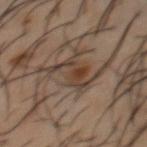workup: catalogued during a skin exam; not biopsied | patient: male, about 55 years old | size: ~5 mm (longest diameter) | image-analysis metrics: a footprint of about 7 mm², an outline eccentricity of about 0.85 (0 = round, 1 = elongated), and two-axis asymmetry of about 0.65; a lesion color around L≈38 a*≈15 b*≈24 in CIELAB, about 9 CIELAB-L* units darker than the surrounding skin, and a normalized lesion–skin contrast near 8; border irregularity of about 9 on a 0–10 scale, a within-lesion color-variation index near 5/10, and a peripheral color-asymmetry measure near 1.5; a classifier nevus-likeness of about 80/100 and a detector confidence of about 90 out of 100 that the crop contains a lesion | image: total-body-photography crop, ~15 mm field of view | illumination: cross-polarized illumination | body site: the chest.